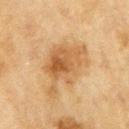{"biopsy_status": "not biopsied; imaged during a skin examination", "patient": {"sex": "male", "age_approx": 70}, "lighting": "cross-polarized", "lesion_size": {"long_diameter_mm_approx": 6.5}, "image": {"source": "total-body photography crop", "field_of_view_mm": 15}, "site": "right upper arm"}